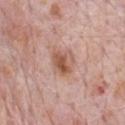Captured during whole-body skin photography for melanoma surveillance; the lesion was not biopsied. The total-body-photography lesion software estimated an outline eccentricity of about 0.55 (0 = round, 1 = elongated) and a shape-asymmetry score of about 0.25 (0 = symmetric). It also reported a mean CIELAB color near L≈56 a*≈22 b*≈28, a lesion–skin lightness drop of about 11, and a lesion-to-skin contrast of about 7.5 (normalized; higher = more distinct). It also reported a border-irregularity index near 2.5/10, internal color variation of about 7.5 on a 0–10 scale, and a peripheral color-asymmetry measure near 2.5. The analysis additionally found a lesion-detection confidence of about 100/100. A male subject aged 68–72. The lesion is located on the front of the torso. Approximately 3.5 mm at its widest. This image is a 15 mm lesion crop taken from a total-body photograph. This is a white-light tile.